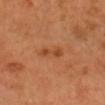workup = imaged on a skin check; not biopsied
anatomic site = the head or neck
illumination = cross-polarized illumination
imaging modality = 15 mm crop, total-body photography
diameter = ≈3 mm
patient = male, aged approximately 50